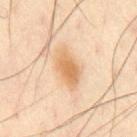Notes:
- lighting · cross-polarized
- imaging modality · 15 mm crop, total-body photography
- image-analysis metrics · an average lesion color of about L≈69 a*≈19 b*≈39 (CIELAB), roughly 10 lightness units darker than nearby skin, and a lesion-to-skin contrast of about 7.5 (normalized; higher = more distinct)
- location · the chest
- diameter · about 5 mm
- patient · male, about 45 years old